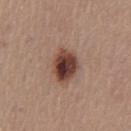The lesion was photographed on a routine skin check and not biopsied; there is no pathology result.
Cropped from a total-body skin-imaging series; the visible field is about 15 mm.
The patient is a male aged 53 to 57.
The lesion is on the mid back.